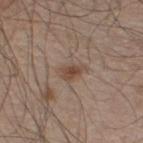No biopsy was performed on this lesion — it was imaged during a full skin examination and was not determined to be concerning.
Automated image analysis of the tile measured a lesion area of about 4.5 mm², a shape eccentricity near 0.75, and a shape-asymmetry score of about 0.3 (0 = symmetric). The software also gave about 9 CIELAB-L* units darker than the surrounding skin. It also reported border irregularity of about 3 on a 0–10 scale.
A 15 mm close-up extracted from a 3D total-body photography capture.
This is a white-light tile.
The patient is a male aged around 60.
Located on the left lower leg.
Longest diameter approximately 3 mm.